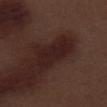Part of a total-body skin-imaging series; this lesion was reviewed on a skin check and was not flagged for biopsy. A male subject about 70 years old. The lesion's longest dimension is about 6 mm. A 15 mm crop from a total-body photograph taken for skin-cancer surveillance. From the right thigh.